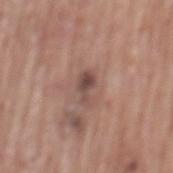  biopsy_status: not biopsied; imaged during a skin examination